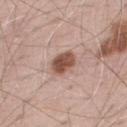Clinical impression:
No biopsy was performed on this lesion — it was imaged during a full skin examination and was not determined to be concerning.
Clinical summary:
Imaged with white-light lighting. Located on the upper back. The total-body-photography lesion software estimated a lesion area of about 6.5 mm², an eccentricity of roughly 0.75, and a symmetry-axis asymmetry near 0.2. And it measured a lesion color around L≈51 a*≈21 b*≈26 in CIELAB, about 15 CIELAB-L* units darker than the surrounding skin, and a lesion-to-skin contrast of about 10.5 (normalized; higher = more distinct). The analysis additionally found a border-irregularity index near 2/10, internal color variation of about 3.5 on a 0–10 scale, and a peripheral color-asymmetry measure near 1. It also reported a nevus-likeness score of about 90/100 and lesion-presence confidence of about 100/100. Cropped from a total-body skin-imaging series; the visible field is about 15 mm. Longest diameter approximately 3.5 mm. A male subject aged approximately 70.